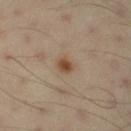No biopsy was performed on this lesion — it was imaged during a full skin examination and was not determined to be concerning. Located on the leg. A close-up tile cropped from a whole-body skin photograph, about 15 mm across. A male patient, approximately 55 years of age. The total-body-photography lesion software estimated an outline eccentricity of about 0.45 (0 = round, 1 = elongated) and a symmetry-axis asymmetry near 0.15. It also reported a border-irregularity index near 1.5/10, a within-lesion color-variation index near 2/10, and peripheral color asymmetry of about 0.5. And it measured an automated nevus-likeness rating near 95 out of 100 and a detector confidence of about 100 out of 100 that the crop contains a lesion.